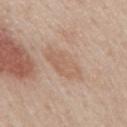  biopsy_status: not biopsied; imaged during a skin examination
  patient:
    sex: male
    age_approx: 60
  image:
    source: total-body photography crop
    field_of_view_mm: 15
  site: back